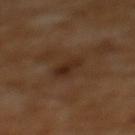<record>
  <biopsy_status>not biopsied; imaged during a skin examination</biopsy_status>
  <image>
    <source>total-body photography crop</source>
    <field_of_view_mm>15</field_of_view_mm>
  </image>
  <lighting>cross-polarized</lighting>
  <lesion_size>
    <long_diameter_mm_approx>3.0</long_diameter_mm_approx>
  </lesion_size>
  <site>upper back</site>
  <automated_metrics>
    <eccentricity>0.7</eccentricity>
    <shape_asymmetry>0.2</shape_asymmetry>
    <cielab_L>28</cielab_L>
    <cielab_a>18</cielab_a>
    <cielab_b>27</cielab_b>
    <vs_skin_contrast_norm>7.0</vs_skin_contrast_norm>
    <color_variation_0_10>4.0</color_variation_0_10>
    <peripheral_color_asymmetry>1.5</peripheral_color_asymmetry>
  </automated_metrics>
  <patient>
    <sex>female</sex>
    <age_approx>55</age_approx>
  </patient>
</record>